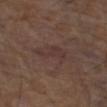Captured during whole-body skin photography for melanoma surveillance; the lesion was not biopsied. Measured at roughly 4 mm in maximum diameter. The total-body-photography lesion software estimated an average lesion color of about L≈34 a*≈17 b*≈19 (CIELAB) and roughly 5 lightness units darker than nearby skin. The analysis additionally found a border-irregularity index near 6/10, a color-variation rating of about 1/10, and peripheral color asymmetry of about 0. The software also gave a nevus-likeness score of about 0/100. From the left thigh. The tile uses white-light illumination. A 15 mm close-up tile from a total-body photography series done for melanoma screening. A male subject approximately 75 years of age.